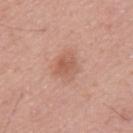Case summary:
• follow-up: no biopsy performed (imaged during a skin exam)
• patient: male, aged 53–57
• diameter: about 3.5 mm
• image: 15 mm crop, total-body photography
• anatomic site: the mid back
• automated lesion analysis: a footprint of about 7 mm², an eccentricity of roughly 0.65, and two-axis asymmetry of about 0.25; an average lesion color of about L≈58 a*≈23 b*≈30 (CIELAB) and a normalized border contrast of about 6; a border-irregularity index near 2/10, a color-variation rating of about 3/10, and a peripheral color-asymmetry measure near 1; a classifier nevus-likeness of about 60/100 and lesion-presence confidence of about 100/100